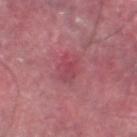No biopsy was performed on this lesion — it was imaged during a full skin examination and was not determined to be concerning. The subject is a male aged around 40. On the left lower leg. The recorded lesion diameter is about 4 mm. Imaged with white-light lighting. A region of skin cropped from a whole-body photographic capture, roughly 15 mm wide.